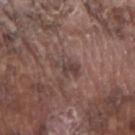<lesion>
<biopsy_status>not biopsied; imaged during a skin examination</biopsy_status>
<image>
  <source>total-body photography crop</source>
  <field_of_view_mm>15</field_of_view_mm>
</image>
<patient>
  <sex>male</sex>
  <age_approx>75</age_approx>
</patient>
<lesion_size>
  <long_diameter_mm_approx>3.0</long_diameter_mm_approx>
</lesion_size>
<site>arm</site>
</lesion>